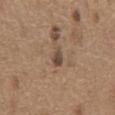Recorded during total-body skin imaging; not selected for excision or biopsy. A male subject, roughly 65 years of age. Located on the abdomen. Cropped from a whole-body photographic skin survey; the tile spans about 15 mm.